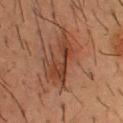A male patient about 55 years old. The lesion's longest dimension is about 5.5 mm. A 15 mm close-up tile from a total-body photography series done for melanoma screening. The lesion is located on the chest. This is a cross-polarized tile. Automated tile analysis of the lesion measured a footprint of about 9 mm² and a shape eccentricity near 0.9. It also reported a lesion color around L≈34 a*≈21 b*≈28 in CIELAB, roughly 9 lightness units darker than nearby skin, and a normalized border contrast of about 8. And it measured a border-irregularity rating of about 6.5/10, a within-lesion color-variation index near 4.5/10, and a peripheral color-asymmetry measure near 2. And it measured an automated nevus-likeness rating near 0 out of 100 and a lesion-detection confidence of about 100/100.